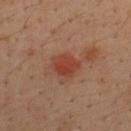Part of a total-body skin-imaging series; this lesion was reviewed on a skin check and was not flagged for biopsy.
Longest diameter approximately 3.5 mm.
Located on the upper back.
Imaged with cross-polarized lighting.
This image is a 15 mm lesion crop taken from a total-body photograph.
The subject is a male aged 33–37.